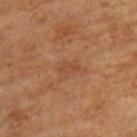This lesion was catalogued during total-body skin photography and was not selected for biopsy.
The lesion is located on the upper back.
Imaged with cross-polarized lighting.
The subject is a male aged 58–62.
Automated image analysis of the tile measured a shape eccentricity near 0.9 and two-axis asymmetry of about 0.35. The analysis additionally found a color-variation rating of about 0/10.
Cropped from a total-body skin-imaging series; the visible field is about 15 mm.